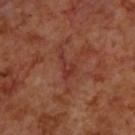No biopsy was performed on this lesion — it was imaged during a full skin examination and was not determined to be concerning.
From the back.
The subject is a male in their 70s.
A region of skin cropped from a whole-body photographic capture, roughly 15 mm wide.